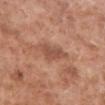Q: Was this lesion biopsied?
A: catalogued during a skin exam; not biopsied
Q: What lighting was used for the tile?
A: white-light illumination
Q: What did automated image analysis measure?
A: a lesion–skin lightness drop of about 9 and a normalized border contrast of about 6.5; a border-irregularity rating of about 3.5/10 and a within-lesion color-variation index near 1.5/10
Q: How was this image acquired?
A: ~15 mm tile from a whole-body skin photo
Q: Where on the body is the lesion?
A: the leg
Q: What is the lesion's diameter?
A: ≈3.5 mm
Q: What are the patient's age and sex?
A: male, aged 78–82